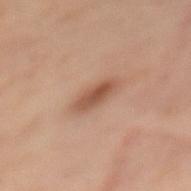Assessment: This lesion was catalogued during total-body skin photography and was not selected for biopsy. Acquisition and patient details: Longest diameter approximately 3.5 mm. A female subject, in their mid- to late 50s. On the back. A 15 mm crop from a total-body photograph taken for skin-cancer surveillance.